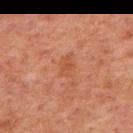The lesion was photographed on a routine skin check and not biopsied; there is no pathology result. A male subject approximately 60 years of age. The lesion is located on the upper back. An algorithmic analysis of the crop reported a nevus-likeness score of about 0/100 and lesion-presence confidence of about 100/100. A 15 mm crop from a total-body photograph taken for skin-cancer surveillance. Imaged with cross-polarized lighting.